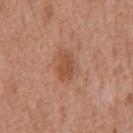The lesion was tiled from a total-body skin photograph and was not biopsied.
The recorded lesion diameter is about 3.5 mm.
Located on the chest.
A male patient, in their mid- to late 70s.
This image is a 15 mm lesion crop taken from a total-body photograph.
The tile uses white-light illumination.